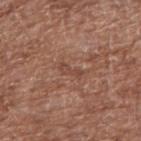biopsy_status: not biopsied; imaged during a skin examination
lesion_size:
  long_diameter_mm_approx: 3.0
automated_metrics:
  area_mm2_approx: 2.5
  eccentricity: 0.95
  border_irregularity_0_10: 4.5
  color_variation_0_10: 0.0
  peripheral_color_asymmetry: 0.0
image:
  source: total-body photography crop
  field_of_view_mm: 15
patient:
  sex: male
  age_approx: 70
site: right upper arm
lighting: white-light